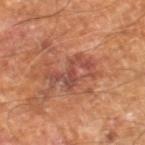biopsy status — catalogued during a skin exam; not biopsied
body site — the right leg
illumination — cross-polarized illumination
patient — male, about 60 years old
image source — ~15 mm tile from a whole-body skin photo